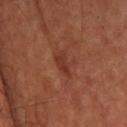Impression:
The lesion was photographed on a routine skin check and not biopsied; there is no pathology result.